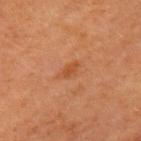workup = no biopsy performed (imaged during a skin exam)
patient = female, aged 53–57
acquisition = ~15 mm tile from a whole-body skin photo
tile lighting = cross-polarized illumination
automated metrics = internal color variation of about 1 on a 0–10 scale and radial color variation of about 0
body site = the left upper arm